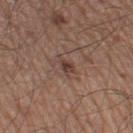follow-up: no biopsy performed (imaged during a skin exam) | imaging modality: 15 mm crop, total-body photography | TBP lesion metrics: a lesion area of about 3.5 mm², an outline eccentricity of about 0.75 (0 = round, 1 = elongated), and a symmetry-axis asymmetry near 0.35; about 9 CIELAB-L* units darker than the surrounding skin and a normalized lesion–skin contrast near 7 | site: the leg | illumination: white-light | size: about 2.5 mm | subject: male, roughly 60 years of age.